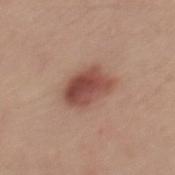Part of a total-body skin-imaging series; this lesion was reviewed on a skin check and was not flagged for biopsy. Automated image analysis of the tile measured a lesion area of about 11 mm², a shape eccentricity near 0.75, and two-axis asymmetry of about 0.2. The analysis additionally found a border-irregularity rating of about 2/10, internal color variation of about 6.5 on a 0–10 scale, and peripheral color asymmetry of about 2.5. And it measured a classifier nevus-likeness of about 100/100 and a detector confidence of about 100 out of 100 that the crop contains a lesion. The lesion's longest dimension is about 4.5 mm. Imaged with white-light lighting. The lesion is on the mid back. A male subject, roughly 30 years of age. This image is a 15 mm lesion crop taken from a total-body photograph.